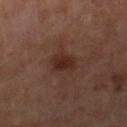Clinical impression:
The lesion was tiled from a total-body skin photograph and was not biopsied.